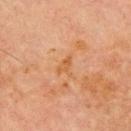| key | value |
|---|---|
| biopsy status | catalogued during a skin exam; not biopsied |
| lighting | cross-polarized |
| automated metrics | an area of roughly 3 mm², an outline eccentricity of about 0.85 (0 = round, 1 = elongated), and a shape-asymmetry score of about 0.4 (0 = symmetric); a mean CIELAB color near L≈48 a*≈21 b*≈35, a lesion–skin lightness drop of about 6, and a normalized border contrast of about 6; border irregularity of about 3.5 on a 0–10 scale, internal color variation of about 2 on a 0–10 scale, and peripheral color asymmetry of about 0.5; lesion-presence confidence of about 100/100 |
| lesion size | ≈2.5 mm |
| acquisition | total-body-photography crop, ~15 mm field of view |
| subject | male, aged 68 to 72 |
| anatomic site | the chest |